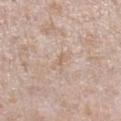The lesion was photographed on a routine skin check and not biopsied; there is no pathology result. From the right lower leg. A female patient about 40 years old. A close-up tile cropped from a whole-body skin photograph, about 15 mm across.